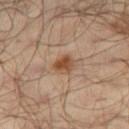notes = no biopsy performed (imaged during a skin exam)
body site = the right thigh
lesion size = ≈2.5 mm
illumination = cross-polarized illumination
acquisition = ~15 mm tile from a whole-body skin photo
patient = male, aged around 65